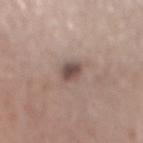<case>
  <biopsy_status>not biopsied; imaged during a skin examination</biopsy_status>
</case>